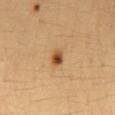biopsy status: total-body-photography surveillance lesion; no biopsy | lesion diameter: about 2 mm | imaging modality: ~15 mm tile from a whole-body skin photo | patient: female, aged around 35 | lighting: cross-polarized | anatomic site: the mid back.